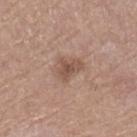{"biopsy_status": "not biopsied; imaged during a skin examination", "lesion_size": {"long_diameter_mm_approx": 3.5}, "image": {"source": "total-body photography crop", "field_of_view_mm": 15}, "automated_metrics": {"border_irregularity_0_10": 4.0, "color_variation_0_10": 2.0, "peripheral_color_asymmetry": 0.5}, "site": "right lower leg", "lighting": "white-light", "patient": {"sex": "female", "age_approx": 55}}